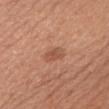Clinical impression:
The lesion was photographed on a routine skin check and not biopsied; there is no pathology result.
Background:
A 15 mm close-up extracted from a 3D total-body photography capture. The total-body-photography lesion software estimated border irregularity of about 2.5 on a 0–10 scale and peripheral color asymmetry of about 1. The analysis additionally found a nevus-likeness score of about 50/100 and lesion-presence confidence of about 100/100. A female patient in their mid-50s. The lesion is located on the head or neck.